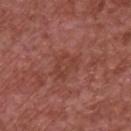A region of skin cropped from a whole-body photographic capture, roughly 15 mm wide. Automated tile analysis of the lesion measured an area of roughly 7.5 mm² and an eccentricity of roughly 0.65. It also reported a color-variation rating of about 2.5/10 and radial color variation of about 1. On the chest. The subject is a male aged around 65.